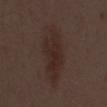biopsy_status: not biopsied; imaged during a skin examination
image:
  source: total-body photography crop
  field_of_view_mm: 15
site: front of the torso
patient:
  sex: male
  age_approx: 55
lesion_size:
  long_diameter_mm_approx: 8.5
lighting: white-light